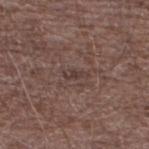| field | value |
|---|---|
| notes | imaged on a skin check; not biopsied |
| location | the leg |
| lesion diameter | ≈2.5 mm |
| subject | male, aged 68 to 72 |
| image | 15 mm crop, total-body photography |